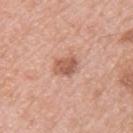lesion size — about 3 mm
anatomic site — the left upper arm
illumination — white-light illumination
patient — male, aged 78–82
image-analysis metrics — a mean CIELAB color near L≈57 a*≈24 b*≈31, about 12 CIELAB-L* units darker than the surrounding skin, and a normalized border contrast of about 8; an automated nevus-likeness rating near 40 out of 100
image — ~15 mm tile from a whole-body skin photo